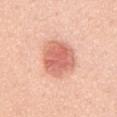Case summary:
- workup: no biopsy performed (imaged during a skin exam)